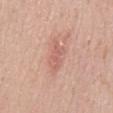| feature | finding |
|---|---|
| biopsy status | no biopsy performed (imaged during a skin exam) |